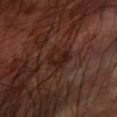| feature | finding |
|---|---|
| workup | total-body-photography surveillance lesion; no biopsy |
| image source | total-body-photography crop, ~15 mm field of view |
| size | ~3 mm (longest diameter) |
| tile lighting | cross-polarized illumination |
| subject | male, aged around 60 |
| location | the left forearm |